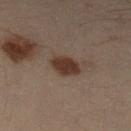Notes:
* follow-up — total-body-photography surveillance lesion; no biopsy
* patient — male, in their mid- to late 50s
* lesion diameter — ~3.5 mm (longest diameter)
* location — the left lower leg
* image source — total-body-photography crop, ~15 mm field of view
* lighting — cross-polarized illumination